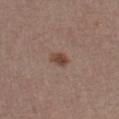| field | value |
|---|---|
| biopsy status | catalogued during a skin exam; not biopsied |
| illumination | white-light |
| image source | total-body-photography crop, ~15 mm field of view |
| anatomic site | the chest |
| subject | male, aged approximately 40 |